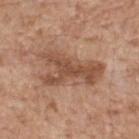follow-up: no biopsy performed (imaged during a skin exam) | image source: ~15 mm tile from a whole-body skin photo | patient: male, aged approximately 60 | lighting: white-light | automated metrics: an area of roughly 19 mm², an eccentricity of roughly 0.9, and a shape-asymmetry score of about 0.5 (0 = symmetric); a border-irregularity index near 6.5/10; an automated nevus-likeness rating near 0 out of 100 and a detector confidence of about 100 out of 100 that the crop contains a lesion | location: the upper back.